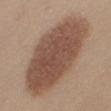Clinical impression: Imaged during a routine full-body skin examination; the lesion was not biopsied and no histopathology is available. Background: A 15 mm close-up extracted from a 3D total-body photography capture. The lesion is on the right thigh. Measured at roughly 12 mm in maximum diameter. A female patient, aged 38 to 42.